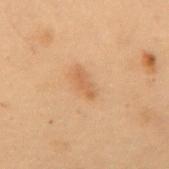Q: Was this lesion biopsied?
A: total-body-photography surveillance lesion; no biopsy
Q: Lesion size?
A: ≈3 mm
Q: What is the anatomic site?
A: the mid back
Q: What did automated image analysis measure?
A: an area of roughly 2.5 mm²; an average lesion color of about L≈49 a*≈19 b*≈32 (CIELAB), a lesion–skin lightness drop of about 7, and a normalized border contrast of about 5.5; a border-irregularity rating of about 5/10, internal color variation of about 0 on a 0–10 scale, and radial color variation of about 0; a classifier nevus-likeness of about 85/100 and a detector confidence of about 100 out of 100 that the crop contains a lesion
Q: What is the imaging modality?
A: ~15 mm tile from a whole-body skin photo
Q: Patient demographics?
A: male, roughly 55 years of age